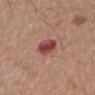anatomic site — the mid back | subject — male, aged around 75 | illumination — white-light | diameter — ≈3 mm | automated metrics — a lesion area of about 6 mm²; a border-irregularity rating of about 2/10, a within-lesion color-variation index near 4.5/10, and a peripheral color-asymmetry measure near 1.5 | acquisition — ~15 mm crop, total-body skin-cancer survey.